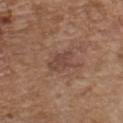  biopsy_status: not biopsied; imaged during a skin examination
  lesion_size:
    long_diameter_mm_approx: 3.5
  site: front of the torso
  image:
    source: total-body photography crop
    field_of_view_mm: 15
  patient:
    sex: male
    age_approx: 75
  lighting: white-light